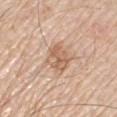Q: Was a biopsy performed?
A: no biopsy performed (imaged during a skin exam)
Q: What is the imaging modality?
A: ~15 mm crop, total-body skin-cancer survey
Q: Where on the body is the lesion?
A: the chest
Q: Patient demographics?
A: male, about 65 years old
Q: How large is the lesion?
A: ≈3.5 mm